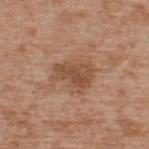workup = imaged on a skin check; not biopsied | patient = male, aged approximately 65 | location = the upper back | imaging modality = ~15 mm tile from a whole-body skin photo | diameter = ≈4.5 mm.